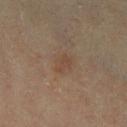follow-up = catalogued during a skin exam; not biopsied | acquisition = ~15 mm crop, total-body skin-cancer survey | anatomic site = the left lower leg | patient = female, aged approximately 55 | lighting = cross-polarized.